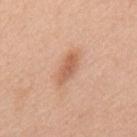{"biopsy_status": "not biopsied; imaged during a skin examination", "automated_metrics": {"area_mm2_approx": 6.0, "eccentricity": 0.9, "shape_asymmetry": 0.3, "cielab_L": 61, "cielab_a": 23, "cielab_b": 33}, "lighting": "white-light", "site": "upper back", "lesion_size": {"long_diameter_mm_approx": 4.0}, "patient": {"sex": "female", "age_approx": 40}, "image": {"source": "total-body photography crop", "field_of_view_mm": 15}}